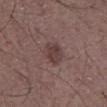| field | value |
|---|---|
| biopsy status | imaged on a skin check; not biopsied |
| subject | male, aged approximately 60 |
| acquisition | 15 mm crop, total-body photography |
| automated metrics | a border-irregularity index near 2/10, internal color variation of about 2.5 on a 0–10 scale, and radial color variation of about 1; a classifier nevus-likeness of about 5/100 |
| site | the chest |
| tile lighting | white-light |